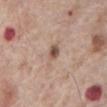biopsy status: catalogued during a skin exam; not biopsied | lighting: white-light illumination | subject: male, about 70 years old | image source: total-body-photography crop, ~15 mm field of view | site: the front of the torso | diameter: ~4 mm (longest diameter).